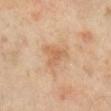The lesion was tiled from a total-body skin photograph and was not biopsied.
This is a cross-polarized tile.
A roughly 15 mm field-of-view crop from a total-body skin photograph.
A female subject, aged around 70.
The total-body-photography lesion software estimated a lesion area of about 6 mm², an outline eccentricity of about 0.65 (0 = round, 1 = elongated), and a shape-asymmetry score of about 0.55 (0 = symmetric). The analysis additionally found an average lesion color of about L≈63 a*≈20 b*≈37 (CIELAB), roughly 8 lightness units darker than nearby skin, and a lesion-to-skin contrast of about 5.5 (normalized; higher = more distinct). And it measured a border-irregularity rating of about 5/10, a color-variation rating of about 2/10, and a peripheral color-asymmetry measure near 0.5.
On the right lower leg.